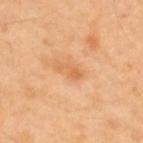site — the upper back
image — 15 mm crop, total-body photography
patient — male, aged approximately 40
automated lesion analysis — a footprint of about 2.5 mm² and two-axis asymmetry of about 0.4; a border-irregularity rating of about 5/10 and a peripheral color-asymmetry measure near 0